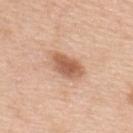Q: Was a biopsy performed?
A: total-body-photography surveillance lesion; no biopsy
Q: Where on the body is the lesion?
A: the back
Q: Patient demographics?
A: male, aged 48–52
Q: What kind of image is this?
A: total-body-photography crop, ~15 mm field of view